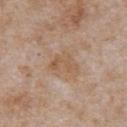biopsy status = catalogued during a skin exam; not biopsied | acquisition = 15 mm crop, total-body photography | anatomic site = the chest | subject = male, approximately 65 years of age | diameter = about 3.5 mm.